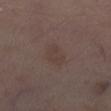This lesion was catalogued during total-body skin photography and was not selected for biopsy.
Cropped from a whole-body photographic skin survey; the tile spans about 15 mm.
From the left lower leg.
Automated tile analysis of the lesion measured a lesion area of about 5.5 mm² and an eccentricity of roughly 0.6. And it measured a nevus-likeness score of about 0/100 and a detector confidence of about 100 out of 100 that the crop contains a lesion.
A male patient, aged around 70.
Longest diameter approximately 3 mm.